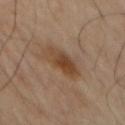<lesion>
<biopsy_status>not biopsied; imaged during a skin examination</biopsy_status>
<image>
  <source>total-body photography crop</source>
  <field_of_view_mm>15</field_of_view_mm>
</image>
<lighting>cross-polarized</lighting>
<patient>
  <sex>male</sex>
  <age_approx>60</age_approx>
</patient>
<lesion_size>
  <long_diameter_mm_approx>4.5</long_diameter_mm_approx>
</lesion_size>
<site>mid back</site>
</lesion>